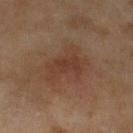workup = total-body-photography surveillance lesion; no biopsy | subject = female, aged around 60 | image source = total-body-photography crop, ~15 mm field of view | body site = the left lower leg.